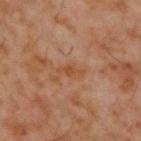| key | value |
|---|---|
| workup | imaged on a skin check; not biopsied |
| acquisition | ~15 mm tile from a whole-body skin photo |
| image-analysis metrics | an eccentricity of roughly 0.9 and a symmetry-axis asymmetry near 0.4; a lesion color around L≈45 a*≈22 b*≈33 in CIELAB, a lesion–skin lightness drop of about 6, and a normalized lesion–skin contrast near 6; a border-irregularity index near 4.5/10 and internal color variation of about 1 on a 0–10 scale |
| location | the upper back |
| patient | male, approximately 60 years of age |